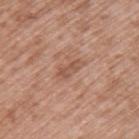Impression:
Recorded during total-body skin imaging; not selected for excision or biopsy.
Context:
A male subject, about 50 years old. The lesion is located on the upper back. Imaged with white-light lighting. About 3 mm across. A 15 mm crop from a total-body photograph taken for skin-cancer surveillance.